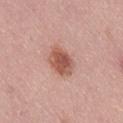{
  "biopsy_status": "not biopsied; imaged during a skin examination",
  "image": {
    "source": "total-body photography crop",
    "field_of_view_mm": 15
  },
  "site": "lower back",
  "patient": {
    "sex": "male",
    "age_approx": 40
  }
}